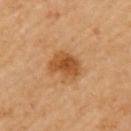<lesion>
<biopsy_status>not biopsied; imaged during a skin examination</biopsy_status>
<site>left upper arm</site>
<patient>
  <sex>female</sex>
  <age_approx>70</age_approx>
</patient>
<image>
  <source>total-body photography crop</source>
  <field_of_view_mm>15</field_of_view_mm>
</image>
<lesion_size>
  <long_diameter_mm_approx>3.5</long_diameter_mm_approx>
</lesion_size>
</lesion>